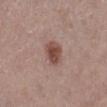follow-up: imaged on a skin check; not biopsied | tile lighting: white-light | subject: female, roughly 50 years of age | diameter: about 3 mm | automated metrics: a lesion color around L≈48 a*≈20 b*≈24 in CIELAB and a normalized border contrast of about 8.5; a color-variation rating of about 3.5/10 and a peripheral color-asymmetry measure near 1 | image: ~15 mm crop, total-body skin-cancer survey | location: the leg.